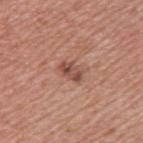Q: Was this lesion biopsied?
A: imaged on a skin check; not biopsied
Q: Patient demographics?
A: female, about 45 years old
Q: How was this image acquired?
A: total-body-photography crop, ~15 mm field of view
Q: Lesion size?
A: about 3 mm
Q: What lighting was used for the tile?
A: white-light illumination
Q: Where on the body is the lesion?
A: the upper back